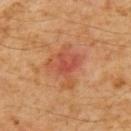Assessment: This lesion was catalogued during total-body skin photography and was not selected for biopsy. Context: The lesion is located on the back. Longest diameter approximately 5.5 mm. A 15 mm close-up tile from a total-body photography series done for melanoma screening. The total-body-photography lesion software estimated a footprint of about 15 mm². The analysis additionally found a lesion color around L≈48 a*≈26 b*≈33 in CIELAB, about 7 CIELAB-L* units darker than the surrounding skin, and a lesion-to-skin contrast of about 6 (normalized; higher = more distinct). And it measured border irregularity of about 5.5 on a 0–10 scale, a within-lesion color-variation index near 5/10, and peripheral color asymmetry of about 1.5. A male patient, in their 60s. Imaged with cross-polarized lighting.